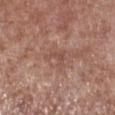follow-up: imaged on a skin check; not biopsied
illumination: white-light
location: the right lower leg
subject: male, aged 53 to 57
acquisition: ~15 mm crop, total-body skin-cancer survey
lesion size: ~2.5 mm (longest diameter)